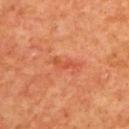Q: How large is the lesion?
A: ~3 mm (longest diameter)
Q: Patient demographics?
A: female, aged 38 to 42
Q: Lesion location?
A: the upper back
Q: Illumination type?
A: cross-polarized illumination
Q: What kind of image is this?
A: ~15 mm tile from a whole-body skin photo